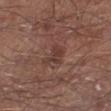| key | value |
|---|---|
| notes | catalogued during a skin exam; not biopsied |
| automated metrics | a lesion color around L≈38 a*≈20 b*≈23 in CIELAB and a normalized lesion–skin contrast near 6 |
| location | the left lower leg |
| patient | male, roughly 55 years of age |
| image | ~15 mm crop, total-body skin-cancer survey |